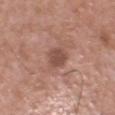Recorded during total-body skin imaging; not selected for excision or biopsy. A 15 mm close-up extracted from a 3D total-body photography capture. Approximately 3 mm at its widest. The lesion is on the head or neck. Captured under white-light illumination. A male patient approximately 50 years of age.